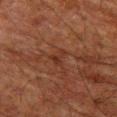Clinical impression: Recorded during total-body skin imaging; not selected for excision or biopsy. Background: The tile uses cross-polarized illumination. On the left thigh. The recorded lesion diameter is about 2.5 mm. A 15 mm crop from a total-body photograph taken for skin-cancer surveillance. The patient is a male aged 78 to 82.